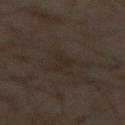Assessment:
Captured during whole-body skin photography for melanoma surveillance; the lesion was not biopsied.
Background:
A 15 mm close-up extracted from a 3D total-body photography capture. Located on the abdomen. A male patient aged around 60.